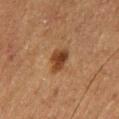follow-up = catalogued during a skin exam; not biopsied
lesion diameter = ~3.5 mm (longest diameter)
body site = the leg
TBP lesion metrics = a lesion area of about 6 mm² and a shape eccentricity near 0.7; a mean CIELAB color near L≈33 a*≈18 b*≈29, a lesion–skin lightness drop of about 11, and a normalized lesion–skin contrast near 10; a color-variation rating of about 3.5/10 and peripheral color asymmetry of about 1; a nevus-likeness score of about 100/100 and lesion-presence confidence of about 100/100
subject = male, aged 73–77
acquisition = ~15 mm tile from a whole-body skin photo
tile lighting = cross-polarized